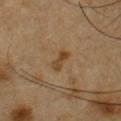follow-up: imaged on a skin check; not biopsied
size: ≈2.5 mm
acquisition: ~15 mm tile from a whole-body skin photo
subject: male, aged 53 to 57
body site: the chest
tile lighting: cross-polarized illumination